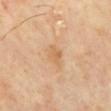<lesion>
  <biopsy_status>not biopsied; imaged during a skin examination</biopsy_status>
  <patient>
    <sex>male</sex>
    <age_approx>65</age_approx>
  </patient>
  <site>mid back</site>
  <image>
    <source>total-body photography crop</source>
    <field_of_view_mm>15</field_of_view_mm>
  </image>
</lesion>